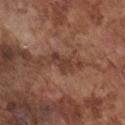The lesion was tiled from a total-body skin photograph and was not biopsied.
The recorded lesion diameter is about 3.5 mm.
A male subject aged around 75.
A 15 mm close-up tile from a total-body photography series done for melanoma screening.
This is a white-light tile.
The lesion is on the chest.